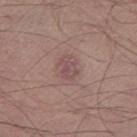Assessment: Part of a total-body skin-imaging series; this lesion was reviewed on a skin check and was not flagged for biopsy. Context: Captured under white-light illumination. The lesion is on the left thigh. A male subject approximately 55 years of age. The recorded lesion diameter is about 3 mm. A roughly 15 mm field-of-view crop from a total-body skin photograph.